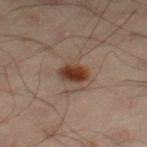Assessment: The lesion was photographed on a routine skin check and not biopsied; there is no pathology result. Image and clinical context: The lesion is on the left leg. A 15 mm close-up extracted from a 3D total-body photography capture. The total-body-photography lesion software estimated a footprint of about 6 mm² and an outline eccentricity of about 0.6 (0 = round, 1 = elongated). A male subject approximately 50 years of age. This is a cross-polarized tile.